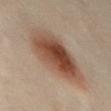A female subject approximately 55 years of age.
Located on the abdomen.
Cropped from a total-body skin-imaging series; the visible field is about 15 mm.
The tile uses cross-polarized illumination.
Automated image analysis of the tile measured an average lesion color of about L≈49 a*≈20 b*≈29 (CIELAB), roughly 14 lightness units darker than nearby skin, and a normalized lesion–skin contrast near 10. The analysis additionally found a nevus-likeness score of about 100/100 and lesion-presence confidence of about 100/100.